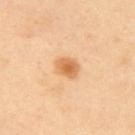biopsy status=catalogued during a skin exam; not biopsied | location=the upper back | subject=female, approximately 40 years of age | acquisition=total-body-photography crop, ~15 mm field of view.